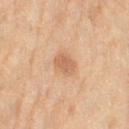Findings:
* workup: no biopsy performed (imaged during a skin exam)
* location: the leg
* lesion size: ~3 mm (longest diameter)
* lighting: cross-polarized illumination
* imaging modality: total-body-photography crop, ~15 mm field of view
* subject: female, in their 80s